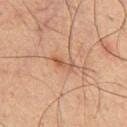notes = total-body-photography surveillance lesion; no biopsy
subject = male, approximately 35 years of age
lesion size = ≈3 mm
acquisition = 15 mm crop, total-body photography
tile lighting = cross-polarized illumination
image-analysis metrics = a color-variation rating of about 0/10 and peripheral color asymmetry of about 0; an automated nevus-likeness rating near 10 out of 100 and a detector confidence of about 100 out of 100 that the crop contains a lesion
site = the chest